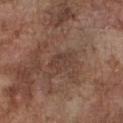image = 15 mm crop, total-body photography | anatomic site = the chest | patient = male, in their mid-70s.